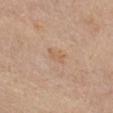The lesion was tiled from a total-body skin photograph and was not biopsied. This image is a 15 mm lesion crop taken from a total-body photograph. A female subject, in their mid- to late 60s. From the chest.